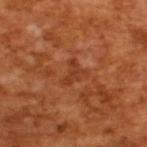– biopsy status — catalogued during a skin exam; not biopsied
– image — 15 mm crop, total-body photography
– patient — male, aged 63 to 67
– TBP lesion metrics — a mean CIELAB color near L≈38 a*≈28 b*≈36, roughly 7 lightness units darker than nearby skin, and a normalized border contrast of about 5.5
– size — ~3 mm (longest diameter)